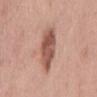workup — no biopsy performed (imaged during a skin exam)
site — the mid back
lesion size — about 5.5 mm
lighting — white-light
subject — male, approximately 70 years of age
image source — total-body-photography crop, ~15 mm field of view
TBP lesion metrics — an area of roughly 13 mm², an eccentricity of roughly 0.85, and a shape-asymmetry score of about 0.15 (0 = symmetric); an average lesion color of about L≈54 a*≈23 b*≈27 (CIELAB), about 14 CIELAB-L* units darker than the surrounding skin, and a normalized border contrast of about 9; radial color variation of about 1.5; an automated nevus-likeness rating near 55 out of 100